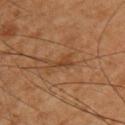notes = catalogued during a skin exam; not biopsied
acquisition = total-body-photography crop, ~15 mm field of view
patient = male, about 60 years old
body site = the right upper arm